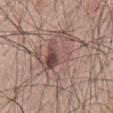| key | value |
|---|---|
| follow-up | total-body-photography surveillance lesion; no biopsy |
| subject | male, roughly 45 years of age |
| lesion diameter | about 9.5 mm |
| acquisition | total-body-photography crop, ~15 mm field of view |
| body site | the mid back |